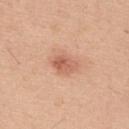<record>
<biopsy_status>not biopsied; imaged during a skin examination</biopsy_status>
<automated_metrics>
  <cielab_L>61</cielab_L>
  <cielab_a>25</cielab_a>
  <cielab_b>32</cielab_b>
  <vs_skin_darker_L>10.0</vs_skin_darker_L>
  <border_irregularity_0_10>2.0</border_irregularity_0_10>
  <color_variation_0_10>4.5</color_variation_0_10>
  <peripheral_color_asymmetry>1.5</peripheral_color_asymmetry>
  <lesion_detection_confidence_0_100>100</lesion_detection_confidence_0_100>
</automated_metrics>
<lighting>white-light</lighting>
<site>upper back</site>
<image>
  <source>total-body photography crop</source>
  <field_of_view_mm>15</field_of_view_mm>
</image>
<patient>
  <sex>male</sex>
  <age_approx>40</age_approx>
</patient>
</record>